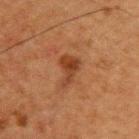| feature | finding |
|---|---|
| location | the upper back |
| imaging modality | 15 mm crop, total-body photography |
| subject | male, aged approximately 50 |